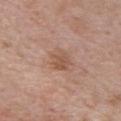Assessment: Part of a total-body skin-imaging series; this lesion was reviewed on a skin check and was not flagged for biopsy. Background: About 3 mm across. A male subject, in their mid- to late 60s. Imaged with white-light lighting. Cropped from a whole-body photographic skin survey; the tile spans about 15 mm. From the chest.